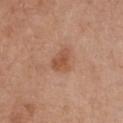The lesion was tiled from a total-body skin photograph and was not biopsied. The lesion is located on the chest. Approximately 2.5 mm at its widest. The lesion-visualizer software estimated roughly 9 lightness units darker than nearby skin and a lesion-to-skin contrast of about 6.5 (normalized; higher = more distinct). The analysis additionally found border irregularity of about 3 on a 0–10 scale, internal color variation of about 2 on a 0–10 scale, and radial color variation of about 0.5. And it measured a nevus-likeness score of about 40/100. Cropped from a total-body skin-imaging series; the visible field is about 15 mm. A female patient aged around 65. The tile uses white-light illumination.